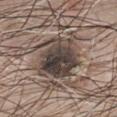Assessment:
Recorded during total-body skin imaging; not selected for excision or biopsy.
Background:
A 15 mm close-up tile from a total-body photography series done for melanoma screening. Automated image analysis of the tile measured a footprint of about 22 mm², a shape eccentricity near 0.65, and a shape-asymmetry score of about 0.2 (0 = symmetric). The software also gave a classifier nevus-likeness of about 5/100 and a lesion-detection confidence of about 5/100. Longest diameter approximately 6 mm. The lesion is on the chest. Imaged with white-light lighting. A male patient, in their 80s.